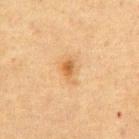Recorded during total-body skin imaging; not selected for excision or biopsy. On the abdomen. The recorded lesion diameter is about 3 mm. A male patient in their mid-70s. A region of skin cropped from a whole-body photographic capture, roughly 15 mm wide. Automated tile analysis of the lesion measured an area of roughly 4.5 mm², an eccentricity of roughly 0.8, and a shape-asymmetry score of about 0.35 (0 = symmetric). It also reported a mean CIELAB color near L≈51 a*≈18 b*≈36, a lesion–skin lightness drop of about 8, and a lesion-to-skin contrast of about 7 (normalized; higher = more distinct). And it measured border irregularity of about 3.5 on a 0–10 scale, a within-lesion color-variation index near 3.5/10, and peripheral color asymmetry of about 1.